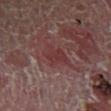notes: imaged on a skin check; not biopsied
image-analysis metrics: an area of roughly 7 mm², a shape eccentricity near 0.55, and a shape-asymmetry score of about 0.45 (0 = symmetric); a border-irregularity rating of about 4.5/10, a within-lesion color-variation index near 2/10, and a peripheral color-asymmetry measure near 0.5; a nevus-likeness score of about 0/100 and a detector confidence of about 55 out of 100 that the crop contains a lesion
diameter: about 3.5 mm
imaging modality: ~15 mm tile from a whole-body skin photo
patient: male, aged 53–57
anatomic site: the left lower leg
tile lighting: cross-polarized illumination